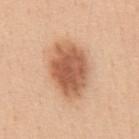Q: Is there a histopathology result?
A: imaged on a skin check; not biopsied
Q: How large is the lesion?
A: ~6.5 mm (longest diameter)
Q: Lesion location?
A: the abdomen
Q: What kind of image is this?
A: 15 mm crop, total-body photography
Q: Who is the patient?
A: male, aged 48 to 52